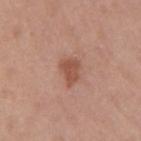Notes:
• biopsy status: no biopsy performed (imaged during a skin exam)
• body site: the right upper arm
• subject: female, approximately 40 years of age
• illumination: white-light
• lesion diameter: about 3 mm
• acquisition: ~15 mm crop, total-body skin-cancer survey